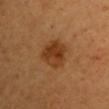Assessment: Part of a total-body skin-imaging series; this lesion was reviewed on a skin check and was not flagged for biopsy. Clinical summary: Longest diameter approximately 4 mm. On the left arm. This image is a 15 mm lesion crop taken from a total-body photograph. A female patient, aged around 45.